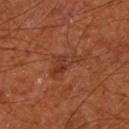| key | value |
|---|---|
| workup | catalogued during a skin exam; not biopsied |
| lesion size | ~4 mm (longest diameter) |
| imaging modality | ~15 mm crop, total-body skin-cancer survey |
| anatomic site | the left lower leg |
| illumination | cross-polarized |
| subject | male, roughly 65 years of age |